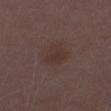Findings:
• body site — the left thigh
• lesion size — ~3.5 mm (longest diameter)
• image — 15 mm crop, total-body photography
• subject — female, in their 30s
• tile lighting — white-light